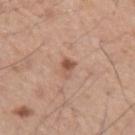Captured during whole-body skin photography for melanoma surveillance; the lesion was not biopsied.
Captured under white-light illumination.
The lesion is located on the left forearm.
The patient is a male aged around 60.
A roughly 15 mm field-of-view crop from a total-body skin photograph.